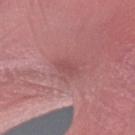notes: imaged on a skin check; not biopsied
patient: female, aged 53 to 57
acquisition: ~15 mm tile from a whole-body skin photo
anatomic site: the right forearm